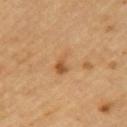Part of a total-body skin-imaging series; this lesion was reviewed on a skin check and was not flagged for biopsy.